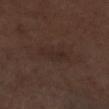{"biopsy_status": "not biopsied; imaged during a skin examination", "image": {"source": "total-body photography crop", "field_of_view_mm": 15}, "patient": {"sex": "male", "age_approx": 70}, "lesion_size": {"long_diameter_mm_approx": 4.0}, "lighting": "white-light", "site": "right lower leg", "automated_metrics": {"area_mm2_approx": 5.5, "eccentricity": 0.95, "shape_asymmetry": 0.3}}